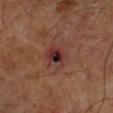Part of a total-body skin-imaging series; this lesion was reviewed on a skin check and was not flagged for biopsy. On the right lower leg. A subject in their mid- to late 60s. This image is a 15 mm lesion crop taken from a total-body photograph.